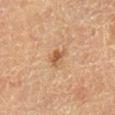Captured during whole-body skin photography for melanoma surveillance; the lesion was not biopsied. A 15 mm close-up tile from a total-body photography series done for melanoma screening. The lesion is on the right lower leg. A male patient approximately 85 years of age or older.